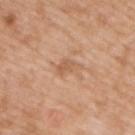Impression:
Recorded during total-body skin imaging; not selected for excision or biopsy.
Acquisition and patient details:
The lesion is located on the upper back. A male subject, aged 48–52. A 15 mm close-up extracted from a 3D total-body photography capture.